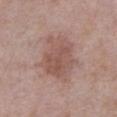This lesion was catalogued during total-body skin photography and was not selected for biopsy. Imaged with white-light lighting. On the abdomen. A lesion tile, about 15 mm wide, cut from a 3D total-body photograph. The subject is a male about 65 years old.